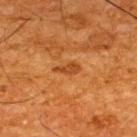follow-up: imaged on a skin check; not biopsied | location: the upper back | lesion size: ≈3 mm | image source: ~15 mm crop, total-body skin-cancer survey | image-analysis metrics: a lesion area of about 3 mm², an eccentricity of roughly 0.9, and a shape-asymmetry score of about 0.45 (0 = symmetric); a border-irregularity rating of about 4.5/10, a within-lesion color-variation index near 1/10, and radial color variation of about 0.5; a nevus-likeness score of about 0/100 and a detector confidence of about 100 out of 100 that the crop contains a lesion | tile lighting: cross-polarized illumination | subject: male, roughly 65 years of age.